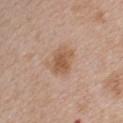No biopsy was performed on this lesion — it was imaged during a full skin examination and was not determined to be concerning. The lesion-visualizer software estimated a lesion area of about 9 mm². And it measured border irregularity of about 2.5 on a 0–10 scale, internal color variation of about 3 on a 0–10 scale, and a peripheral color-asymmetry measure near 0.5. Located on the back. A lesion tile, about 15 mm wide, cut from a 3D total-body photograph. The subject is a female aged approximately 45.